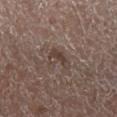  patient:
    sex: female
    age_approx: 70
  site: right lower leg
  lesion_size:
    long_diameter_mm_approx: 3.0
  image:
    source: total-body photography crop
    field_of_view_mm: 15
  lighting: white-light